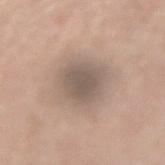biopsy_status: not biopsied; imaged during a skin examination
patient:
  sex: female
  age_approx: 50
image:
  source: total-body photography crop
  field_of_view_mm: 15
site: right forearm
automated_metrics:
  cielab_L: 55
  cielab_a: 12
  cielab_b: 23
  vs_skin_darker_L: 10.0
  vs_skin_contrast_norm: 7.0
lighting: white-light
lesion_size:
  long_diameter_mm_approx: 4.5